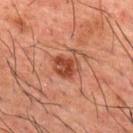Notes:
– follow-up · total-body-photography surveillance lesion; no biopsy
– body site · the back
– image · ~15 mm tile from a whole-body skin photo
– subject · male, in their 50s